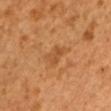{"biopsy_status": "not biopsied; imaged during a skin examination", "site": "arm", "patient": {"sex": "male", "age_approx": 50}, "lighting": "cross-polarized", "lesion_size": {"long_diameter_mm_approx": 2.5}, "image": {"source": "total-body photography crop", "field_of_view_mm": 15}}